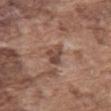| feature | finding |
|---|---|
| notes | total-body-photography surveillance lesion; no biopsy |
| tile lighting | white-light |
| lesion size | ≈3 mm |
| subject | male, aged approximately 75 |
| acquisition | ~15 mm crop, total-body skin-cancer survey |
| site | the mid back |